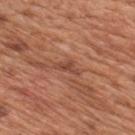Captured during whole-body skin photography for melanoma surveillance; the lesion was not biopsied. Cropped from a total-body skin-imaging series; the visible field is about 15 mm. The lesion is located on the mid back. A male patient, about 65 years old. This is a white-light tile.